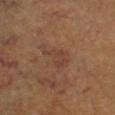Part of a total-body skin-imaging series; this lesion was reviewed on a skin check and was not flagged for biopsy.